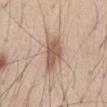| key | value |
|---|---|
| notes | no biopsy performed (imaged during a skin exam) |
| site | the abdomen |
| image | total-body-photography crop, ~15 mm field of view |
| patient | male, aged 43 to 47 |